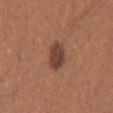Assessment:
Imaged during a routine full-body skin examination; the lesion was not biopsied and no histopathology is available.
Context:
A female patient, in their mid-20s. Captured under white-light illumination. A lesion tile, about 15 mm wide, cut from a 3D total-body photograph. Automated tile analysis of the lesion measured an area of roughly 7.5 mm², an eccentricity of roughly 0.8, and a shape-asymmetry score of about 0.15 (0 = symmetric). And it measured a classifier nevus-likeness of about 80/100. About 4 mm across. From the mid back.